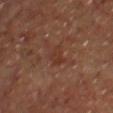<lesion>
  <biopsy_status>not biopsied; imaged during a skin examination</biopsy_status>
</lesion>